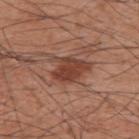Imaged during a routine full-body skin examination; the lesion was not biopsied and no histopathology is available. Measured at roughly 5 mm in maximum diameter. The subject is a male aged 58–62. The lesion is located on the upper back. This is a white-light tile. Cropped from a total-body skin-imaging series; the visible field is about 15 mm.